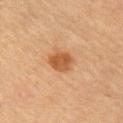Findings:
• notes — no biopsy performed (imaged during a skin exam)
• diameter — ≈3 mm
• body site — the left upper arm
• illumination — cross-polarized illumination
• image source — ~15 mm tile from a whole-body skin photo
• patient — male, aged around 65